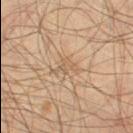Recorded during total-body skin imaging; not selected for excision or biopsy.
A region of skin cropped from a whole-body photographic capture, roughly 15 mm wide.
The lesion is located on the left thigh.
Automated image analysis of the tile measured an area of roughly 3 mm², an eccentricity of roughly 0.75, and a shape-asymmetry score of about 0.55 (0 = symmetric). The software also gave a classifier nevus-likeness of about 0/100 and a lesion-detection confidence of about 75/100.
Approximately 3 mm at its widest.
The patient is a male in their mid-50s.